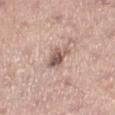biopsy_status: not biopsied; imaged during a skin examination
lighting: white-light
image:
  source: total-body photography crop
  field_of_view_mm: 15
site: left lower leg
lesion_size:
  long_diameter_mm_approx: 3.0
patient:
  sex: female
  age_approx: 65